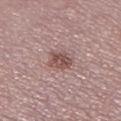Case summary:
* workup: no biopsy performed (imaged during a skin exam)
* image: total-body-photography crop, ~15 mm field of view
* automated metrics: a lesion area of about 6 mm², a shape eccentricity near 0.5, and a symmetry-axis asymmetry near 0.25; a mean CIELAB color near L≈51 a*≈20 b*≈21, about 11 CIELAB-L* units darker than the surrounding skin, and a lesion-to-skin contrast of about 7.5 (normalized; higher = more distinct); a border-irregularity index near 2.5/10, a color-variation rating of about 4/10, and peripheral color asymmetry of about 1.5
* illumination: white-light
* anatomic site: the left lower leg
* patient: female, aged 53–57
* lesion size: ~3 mm (longest diameter)